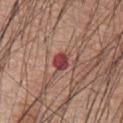The lesion was photographed on a routine skin check and not biopsied; there is no pathology result.
The lesion is on the chest.
A 15 mm close-up tile from a total-body photography series done for melanoma screening.
A male patient aged 58–62.